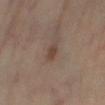Background: This is a cross-polarized tile. An algorithmic analysis of the crop reported a lesion area of about 2.5 mm², an eccentricity of roughly 0.8, and a symmetry-axis asymmetry near 0.25. The analysis additionally found a lesion color around L≈40 a*≈16 b*≈25 in CIELAB and a lesion-to-skin contrast of about 7.5 (normalized; higher = more distinct). The analysis additionally found a nevus-likeness score of about 45/100 and a detector confidence of about 100 out of 100 that the crop contains a lesion. Located on the left lower leg. Measured at roughly 2 mm in maximum diameter. A region of skin cropped from a whole-body photographic capture, roughly 15 mm wide. A female patient, approximately 65 years of age.